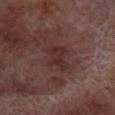The lesion was tiled from a total-body skin photograph and was not biopsied. Approximately 3.5 mm at its widest. An algorithmic analysis of the crop reported a footprint of about 6.5 mm², an outline eccentricity of about 0.8 (0 = round, 1 = elongated), and a shape-asymmetry score of about 0.4 (0 = symmetric). The software also gave a border-irregularity rating of about 5/10 and a within-lesion color-variation index near 2.5/10. And it measured lesion-presence confidence of about 100/100. A roughly 15 mm field-of-view crop from a total-body skin photograph. Captured under cross-polarized illumination. Located on the left lower leg. The subject is a male approximately 70 years of age.